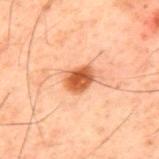Case summary:
* follow-up — total-body-photography surveillance lesion; no biopsy
* diameter — ≈3 mm
* subject — male, approximately 60 years of age
* image source — ~15 mm tile from a whole-body skin photo
* automated metrics — a mean CIELAB color near L≈45 a*≈25 b*≈34 and roughly 14 lightness units darker than nearby skin; a classifier nevus-likeness of about 100/100
* site — the upper back
* illumination — cross-polarized illumination